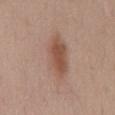The lesion was photographed on a routine skin check and not biopsied; there is no pathology result.
Longest diameter approximately 7.5 mm.
A 15 mm crop from a total-body photograph taken for skin-cancer surveillance.
The tile uses white-light illumination.
A male subject in their 60s.
The lesion is located on the chest.